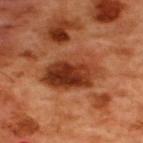workup = total-body-photography surveillance lesion; no biopsy
diameter = ≈7 mm
automated lesion analysis = a border-irregularity rating of about 2/10, a within-lesion color-variation index near 9/10, and radial color variation of about 4
acquisition = ~15 mm crop, total-body skin-cancer survey
location = the back
tile lighting = cross-polarized illumination
subject = male, aged approximately 50